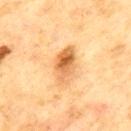Captured during whole-body skin photography for melanoma surveillance; the lesion was not biopsied. This image is a 15 mm lesion crop taken from a total-body photograph. The lesion's longest dimension is about 4.5 mm. The lesion is located on the mid back. A male patient approximately 70 years of age.